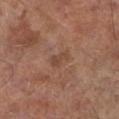Recorded during total-body skin imaging; not selected for excision or biopsy. A male subject, about 70 years old. Cropped from a whole-body photographic skin survey; the tile spans about 15 mm. The lesion is located on the left lower leg.